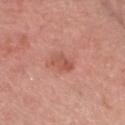Clinical impression:
The lesion was tiled from a total-body skin photograph and was not biopsied.
Acquisition and patient details:
Located on the head or neck. The subject is a male approximately 80 years of age. A 15 mm crop from a total-body photograph taken for skin-cancer surveillance.